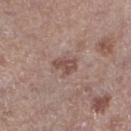Impression:
Imaged during a routine full-body skin examination; the lesion was not biopsied and no histopathology is available.
Acquisition and patient details:
The recorded lesion diameter is about 3 mm. This is a white-light tile. The lesion-visualizer software estimated a footprint of about 4.5 mm², an outline eccentricity of about 0.75 (0 = round, 1 = elongated), and a symmetry-axis asymmetry near 0.35. The analysis additionally found an average lesion color of about L≈49 a*≈19 b*≈23 (CIELAB) and a lesion-to-skin contrast of about 7 (normalized; higher = more distinct). From the left lower leg. The subject is a male aged 78 to 82. A lesion tile, about 15 mm wide, cut from a 3D total-body photograph.